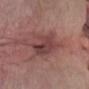follow-up = no biopsy performed (imaged during a skin exam)
lesion diameter = ~7.5 mm (longest diameter)
lighting = white-light
patient = male, aged 58 to 62
acquisition = ~15 mm tile from a whole-body skin photo
location = the abdomen
automated lesion analysis = a lesion area of about 16 mm² and a shape eccentricity near 0.9; a lesion color around L≈43 a*≈23 b*≈20 in CIELAB, about 10 CIELAB-L* units darker than the surrounding skin, and a normalized border contrast of about 8.5; internal color variation of about 7 on a 0–10 scale and peripheral color asymmetry of about 2.5; a classifier nevus-likeness of about 0/100 and lesion-presence confidence of about 75/100